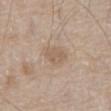Part of a total-body skin-imaging series; this lesion was reviewed on a skin check and was not flagged for biopsy.
Measured at roughly 3 mm in maximum diameter.
A region of skin cropped from a whole-body photographic capture, roughly 15 mm wide.
The total-body-photography lesion software estimated a color-variation rating of about 1.5/10 and radial color variation of about 0.5.
The patient is a male aged around 80.
The lesion is located on the abdomen.